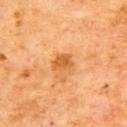Assessment:
This lesion was catalogued during total-body skin photography and was not selected for biopsy.
Image and clinical context:
A 15 mm crop from a total-body photograph taken for skin-cancer surveillance. From the upper back. A male patient roughly 60 years of age.